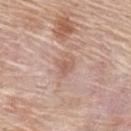Q: Is there a histopathology result?
A: total-body-photography surveillance lesion; no biopsy
Q: Automated lesion metrics?
A: a lesion area of about 4.5 mm², an eccentricity of roughly 0.65, and a shape-asymmetry score of about 0.3 (0 = symmetric); a nevus-likeness score of about 0/100 and a detector confidence of about 100 out of 100 that the crop contains a lesion
Q: Where on the body is the lesion?
A: the upper back
Q: What is the imaging modality?
A: 15 mm crop, total-body photography
Q: What lighting was used for the tile?
A: white-light
Q: Who is the patient?
A: female, in their mid-60s
Q: How large is the lesion?
A: ~2.5 mm (longest diameter)